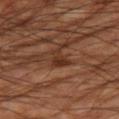Imaged during a routine full-body skin examination; the lesion was not biopsied and no histopathology is available.
The recorded lesion diameter is about 3 mm.
A roughly 15 mm field-of-view crop from a total-body skin photograph.
A male subject aged 58–62.
From the right thigh.
Captured under cross-polarized illumination.